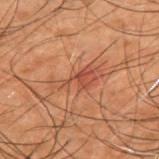Case summary:
• workup — total-body-photography surveillance lesion; no biopsy
• TBP lesion metrics — border irregularity of about 6.5 on a 0–10 scale, a color-variation rating of about 2.5/10, and radial color variation of about 1
• size — about 5.5 mm
• subject — male, aged approximately 50
• anatomic site — the upper back
• image source — total-body-photography crop, ~15 mm field of view
• lighting — cross-polarized illumination